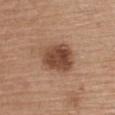follow-up=no biopsy performed (imaged during a skin exam).